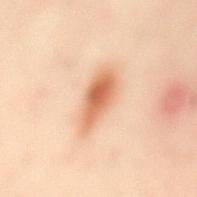Clinical impression: Recorded during total-body skin imaging; not selected for excision or biopsy. Image and clinical context: A female patient, aged approximately 40. On the mid back. Captured under cross-polarized illumination. A region of skin cropped from a whole-body photographic capture, roughly 15 mm wide. Automated image analysis of the tile measured an outline eccentricity of about 0.9 (0 = round, 1 = elongated) and a symmetry-axis asymmetry near 0.25. It also reported a mean CIELAB color near L≈65 a*≈24 b*≈37. The recorded lesion diameter is about 5.5 mm.